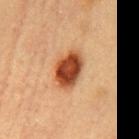Findings:
• follow-up · no biopsy performed (imaged during a skin exam)
• location · the mid back
• patient · female, aged 58–62
• lesion size · ~4.5 mm (longest diameter)
• imaging modality · ~15 mm tile from a whole-body skin photo
• tile lighting · cross-polarized
• automated metrics · a mean CIELAB color near L≈43 a*≈26 b*≈35, a lesion–skin lightness drop of about 18, and a lesion-to-skin contrast of about 13 (normalized; higher = more distinct)